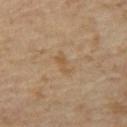Findings:
– notes · imaged on a skin check; not biopsied
– illumination · cross-polarized illumination
– imaging modality · ~15 mm crop, total-body skin-cancer survey
– TBP lesion metrics · an area of roughly 2.5 mm², a shape eccentricity near 0.9, and a symmetry-axis asymmetry near 0.4; an automated nevus-likeness rating near 0 out of 100
– patient · male, in their mid-60s
– diameter · ~2.5 mm (longest diameter)